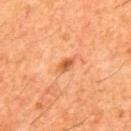Q: Was this lesion biopsied?
A: imaged on a skin check; not biopsied
Q: Illumination type?
A: cross-polarized illumination
Q: What kind of image is this?
A: ~15 mm tile from a whole-body skin photo
Q: Lesion location?
A: the mid back
Q: Lesion size?
A: ≈2.5 mm
Q: Patient demographics?
A: male, aged 58 to 62
Q: What did automated image analysis measure?
A: a nevus-likeness score of about 55/100